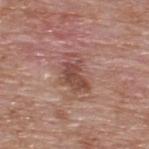notes = imaged on a skin check; not biopsied
image = total-body-photography crop, ~15 mm field of view
body site = the upper back
subject = male, about 60 years old